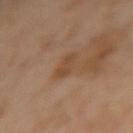Imaged during a routine full-body skin examination; the lesion was not biopsied and no histopathology is available.
Automated image analysis of the tile measured an average lesion color of about L≈47 a*≈19 b*≈33 (CIELAB), about 7 CIELAB-L* units darker than the surrounding skin, and a normalized border contrast of about 6. It also reported a color-variation rating of about 1.5/10 and a peripheral color-asymmetry measure near 0.5.
The subject is a female approximately 55 years of age.
The lesion is located on the arm.
A close-up tile cropped from a whole-body skin photograph, about 15 mm across.